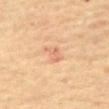Part of a total-body skin-imaging series; this lesion was reviewed on a skin check and was not flagged for biopsy. A male patient, aged approximately 65. A region of skin cropped from a whole-body photographic capture, roughly 15 mm wide. The lesion's longest dimension is about 3 mm. On the abdomen.